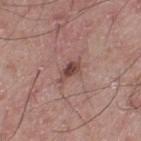Recorded during total-body skin imaging; not selected for excision or biopsy. Measured at roughly 3 mm in maximum diameter. The total-body-photography lesion software estimated a shape eccentricity near 0.85 and two-axis asymmetry of about 0.35. The software also gave an average lesion color of about L≈45 a*≈21 b*≈22 (CIELAB), about 12 CIELAB-L* units darker than the surrounding skin, and a lesion-to-skin contrast of about 8.5 (normalized; higher = more distinct). It also reported a classifier nevus-likeness of about 75/100 and a detector confidence of about 100 out of 100 that the crop contains a lesion. A male patient, about 50 years old. A close-up tile cropped from a whole-body skin photograph, about 15 mm across. The lesion is located on the left thigh.